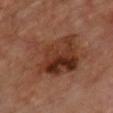A male patient, in their mid-50s. About 8.5 mm across. A lesion tile, about 15 mm wide, cut from a 3D total-body photograph. Captured under cross-polarized illumination. The lesion is located on the front of the torso. The total-body-photography lesion software estimated a lesion color around L≈36 a*≈22 b*≈28 in CIELAB, about 9 CIELAB-L* units darker than the surrounding skin, and a lesion-to-skin contrast of about 8 (normalized; higher = more distinct). The software also gave a border-irregularity index near 4/10, internal color variation of about 8.5 on a 0–10 scale, and peripheral color asymmetry of about 3. And it measured a nevus-likeness score of about 35/100 and a lesion-detection confidence of about 100/100.